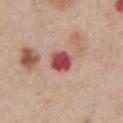About 3 mm across.
A male subject aged 58 to 62.
Automated image analysis of the tile measured an eccentricity of roughly 0.4 and a shape-asymmetry score of about 0.15 (0 = symmetric). The analysis additionally found a mean CIELAB color near L≈50 a*≈34 b*≈25, about 17 CIELAB-L* units darker than the surrounding skin, and a lesion-to-skin contrast of about 11.5 (normalized; higher = more distinct). The analysis additionally found a border-irregularity rating of about 1.5/10, a within-lesion color-variation index near 4/10, and peripheral color asymmetry of about 1. And it measured a nevus-likeness score of about 0/100 and a detector confidence of about 100 out of 100 that the crop contains a lesion.
This image is a 15 mm lesion crop taken from a total-body photograph.
Captured under white-light illumination.
Located on the chest.